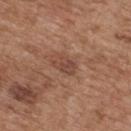Impression: Captured during whole-body skin photography for melanoma surveillance; the lesion was not biopsied.